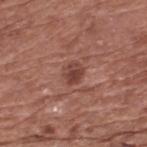Impression: This lesion was catalogued during total-body skin photography and was not selected for biopsy. Background: From the upper back. Automated tile analysis of the lesion measured a mean CIELAB color near L≈42 a*≈24 b*≈24, roughly 10 lightness units darker than nearby skin, and a lesion-to-skin contrast of about 8 (normalized; higher = more distinct). And it measured a border-irregularity rating of about 2/10, internal color variation of about 3.5 on a 0–10 scale, and peripheral color asymmetry of about 1.5. The software also gave a classifier nevus-likeness of about 60/100 and a detector confidence of about 100 out of 100 that the crop contains a lesion. Captured under white-light illumination. The patient is a male about 75 years old. The lesion's longest dimension is about 2.5 mm. A roughly 15 mm field-of-view crop from a total-body skin photograph.